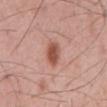- notes — imaged on a skin check; not biopsied
- anatomic site — the mid back
- subject — male, aged 53–57
- diameter — ~3 mm (longest diameter)
- imaging modality — total-body-photography crop, ~15 mm field of view
- tile lighting — white-light illumination